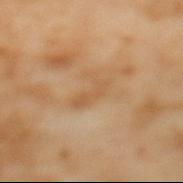Part of a total-body skin-imaging series; this lesion was reviewed on a skin check and was not flagged for biopsy.